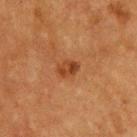Impression: Captured during whole-body skin photography for melanoma surveillance; the lesion was not biopsied. Background: This is a cross-polarized tile. Approximately 2.5 mm at its widest. Cropped from a whole-body photographic skin survey; the tile spans about 15 mm. A male patient, in their mid-70s. Located on the upper back.